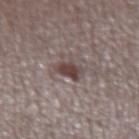* biopsy status — imaged on a skin check; not biopsied
* automated lesion analysis — a lesion area of about 5.5 mm², an outline eccentricity of about 0.55 (0 = round, 1 = elongated), and a shape-asymmetry score of about 0.25 (0 = symmetric); a border-irregularity rating of about 2.5/10, a color-variation rating of about 3/10, and radial color variation of about 1; lesion-presence confidence of about 65/100
* image — ~15 mm tile from a whole-body skin photo
* size — ~3 mm (longest diameter)
* illumination — white-light illumination
* site — the right forearm
* patient — female, aged around 55